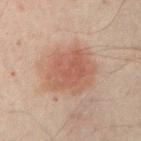biopsy status — no biopsy performed (imaged during a skin exam) | body site — the right upper arm | subject — male, aged 48 to 52 | image-analysis metrics — a lesion area of about 23 mm², a shape eccentricity near 0.35, and a symmetry-axis asymmetry near 0.2; a lesion color around L≈51 a*≈20 b*≈27 in CIELAB, a lesion–skin lightness drop of about 8, and a lesion-to-skin contrast of about 6.5 (normalized; higher = more distinct); a border-irregularity index near 2.5/10, a color-variation rating of about 3.5/10, and radial color variation of about 1; a classifier nevus-likeness of about 95/100 and a lesion-detection confidence of about 100/100 | image source — total-body-photography crop, ~15 mm field of view | lighting — cross-polarized illumination.